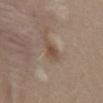{
  "biopsy_status": "not biopsied; imaged during a skin examination",
  "image": {
    "source": "total-body photography crop",
    "field_of_view_mm": 15
  },
  "patient": {
    "sex": "male",
    "age_approx": 30
  },
  "site": "mid back"
}